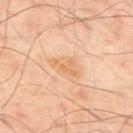biopsy status = imaged on a skin check; not biopsied
diameter = about 4 mm
location = the upper back
imaging modality = ~15 mm crop, total-body skin-cancer survey
lighting = cross-polarized
patient = male, aged 48 to 52